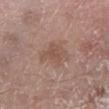The lesion was photographed on a routine skin check and not biopsied; there is no pathology result.
A 15 mm close-up extracted from a 3D total-body photography capture.
The subject is a male aged approximately 80.
On the right lower leg.
Imaged with white-light lighting.
Automated image analysis of the tile measured a lesion area of about 7 mm² and two-axis asymmetry of about 0.35. And it measured about 7 CIELAB-L* units darker than the surrounding skin and a lesion-to-skin contrast of about 5.5 (normalized; higher = more distinct). The software also gave border irregularity of about 4 on a 0–10 scale, internal color variation of about 2 on a 0–10 scale, and peripheral color asymmetry of about 0.5.
Longest diameter approximately 4 mm.